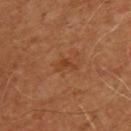Notes:
• biopsy status: imaged on a skin check; not biopsied
• location: the upper back
• tile lighting: cross-polarized illumination
• size: about 2.5 mm
• image source: 15 mm crop, total-body photography
• patient: male, aged approximately 50